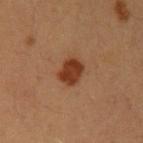* biopsy status — catalogued during a skin exam; not biopsied
* subject — male, in their 40s
* automated lesion analysis — an area of roughly 7 mm², a shape eccentricity near 0.35, and a shape-asymmetry score of about 0.15 (0 = symmetric); a lesion color around L≈33 a*≈23 b*≈30 in CIELAB and a normalized border contrast of about 10.5; a border-irregularity index near 1.5/10
* lesion size — ≈3 mm
* body site — the left upper arm
* lighting — cross-polarized illumination
* acquisition — total-body-photography crop, ~15 mm field of view